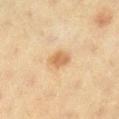biopsy_status: not biopsied; imaged during a skin examination